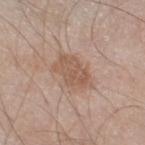biopsy status: total-body-photography surveillance lesion; no biopsy | image source: ~15 mm crop, total-body skin-cancer survey | illumination: white-light | automated lesion analysis: a lesion area of about 10 mm² and a shape eccentricity near 0.75; a border-irregularity rating of about 3/10, internal color variation of about 3 on a 0–10 scale, and a peripheral color-asymmetry measure near 1 | body site: the right thigh | subject: male, roughly 60 years of age.